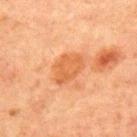biopsy status=no biopsy performed (imaged during a skin exam)
tile lighting=cross-polarized
lesion size=about 4 mm
anatomic site=the front of the torso
imaging modality=15 mm crop, total-body photography
subject=male, aged approximately 65